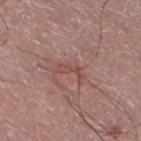notes = no biopsy performed (imaged during a skin exam)
image = ~15 mm tile from a whole-body skin photo
diameter = ~3 mm (longest diameter)
patient = male, in their mid- to late 70s
tile lighting = white-light illumination
anatomic site = the left lower leg